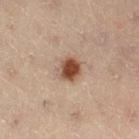Impression: Part of a total-body skin-imaging series; this lesion was reviewed on a skin check and was not flagged for biopsy. Clinical summary: Located on the right thigh. This is a cross-polarized tile. The recorded lesion diameter is about 3 mm. The lesion-visualizer software estimated an average lesion color of about L≈43 a*≈18 b*≈27 (CIELAB), roughly 14 lightness units darker than nearby skin, and a normalized lesion–skin contrast near 11. It also reported a border-irregularity index near 1.5/10, internal color variation of about 4.5 on a 0–10 scale, and peripheral color asymmetry of about 1. The subject is a male about 50 years old. Cropped from a whole-body photographic skin survey; the tile spans about 15 mm.